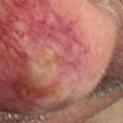Impression:
No biopsy was performed on this lesion — it was imaged during a full skin examination and was not determined to be concerning.
Clinical summary:
A 15 mm close-up tile from a total-body photography series done for melanoma screening. The lesion's longest dimension is about 1.5 mm. Captured under cross-polarized illumination. A male subject about 60 years old. The lesion is on the head or neck.